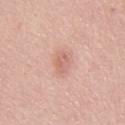Impression:
Recorded during total-body skin imaging; not selected for excision or biopsy.
Context:
This is a white-light tile. From the abdomen. The subject is a male aged 63 to 67. A close-up tile cropped from a whole-body skin photograph, about 15 mm across. The lesion's longest dimension is about 2.5 mm.